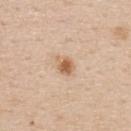workup: total-body-photography surveillance lesion; no biopsy
body site: the back
lesion diameter: ~2.5 mm (longest diameter)
patient: female, aged 43 to 47
acquisition: ~15 mm crop, total-body skin-cancer survey